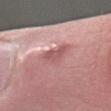Q: Was this lesion biopsied?
A: total-body-photography surveillance lesion; no biopsy
Q: What are the patient's age and sex?
A: male, about 20 years old
Q: What is the anatomic site?
A: the arm
Q: How was this image acquired?
A: total-body-photography crop, ~15 mm field of view
Q: What is the lesion's diameter?
A: ~3.5 mm (longest diameter)
Q: Illumination type?
A: white-light